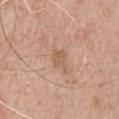This lesion was catalogued during total-body skin photography and was not selected for biopsy.
On the chest.
This image is a 15 mm lesion crop taken from a total-body photograph.
This is a white-light tile.
An algorithmic analysis of the crop reported a shape eccentricity near 0.7. The software also gave a mean CIELAB color near L≈59 a*≈20 b*≈31, roughly 7 lightness units darker than nearby skin, and a normalized lesion–skin contrast near 5.5. The analysis additionally found internal color variation of about 1.5 on a 0–10 scale. The software also gave a nevus-likeness score of about 0/100.
The subject is a male aged around 80.
Measured at roughly 2.5 mm in maximum diameter.